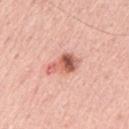Clinical summary: The tile uses white-light illumination. A close-up tile cropped from a whole-body skin photograph, about 15 mm across. The subject is a male about 65 years old. The lesion is on the left upper arm. Longest diameter approximately 3.5 mm.